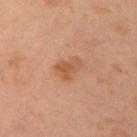Imaged during a routine full-body skin examination; the lesion was not biopsied and no histopathology is available. The lesion is on the left upper arm. Captured under cross-polarized illumination. A female patient, approximately 55 years of age. Automated image analysis of the tile measured a mean CIELAB color near L≈45 a*≈20 b*≈30 and a lesion-to-skin contrast of about 6 (normalized; higher = more distinct). The software also gave a border-irregularity rating of about 3.5/10, a color-variation rating of about 3/10, and peripheral color asymmetry of about 1. Measured at roughly 3 mm in maximum diameter. A lesion tile, about 15 mm wide, cut from a 3D total-body photograph.